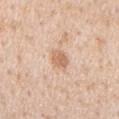Recorded during total-body skin imaging; not selected for excision or biopsy. The lesion is located on the mid back. The subject is a male about 65 years old. A 15 mm close-up extracted from a 3D total-body photography capture.